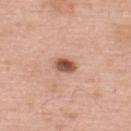workup: total-body-photography surveillance lesion; no biopsy | imaging modality: total-body-photography crop, ~15 mm field of view | lighting: white-light | lesion diameter: about 2.5 mm | patient: male, about 60 years old | location: the upper back.